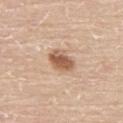  biopsy_status: not biopsied; imaged during a skin examination
  site: upper back
  lighting: white-light
  lesion_size:
    long_diameter_mm_approx: 3.5
  automated_metrics:
    cielab_L: 58
    cielab_a: 20
    cielab_b: 32
    vs_skin_darker_L: 14.0
    vs_skin_contrast_norm: 9.0
    border_irregularity_0_10: 2.0
    color_variation_0_10: 4.5
    peripheral_color_asymmetry: 1.5
  patient:
    sex: male
    age_approx: 60
  image:
    source: total-body photography crop
    field_of_view_mm: 15